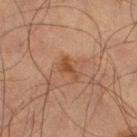Cropped from a total-body skin-imaging series; the visible field is about 15 mm.
Located on the left thigh.
A male patient, aged 63–67.